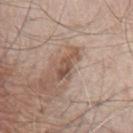Imaged during a routine full-body skin examination; the lesion was not biopsied and no histopathology is available.
This is a white-light tile.
Cropped from a total-body skin-imaging series; the visible field is about 15 mm.
Automated tile analysis of the lesion measured a classifier nevus-likeness of about 10/100.
The recorded lesion diameter is about 4 mm.
The patient is a male aged around 65.
The lesion is located on the chest.